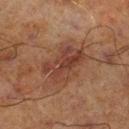biopsy_status: not biopsied; imaged during a skin examination
patient:
  sex: male
  age_approx: 70
site: right lower leg
image:
  source: total-body photography crop
  field_of_view_mm: 15
lesion_size:
  long_diameter_mm_approx: 5.5
automated_metrics:
  cielab_L: 33
  cielab_a: 19
  cielab_b: 24
  vs_skin_darker_L: 7.0
  vs_skin_contrast_norm: 7.0
  nevus_likeness_0_100: 10
  lesion_detection_confidence_0_100: 100